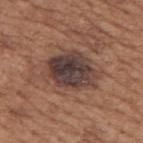Captured during whole-body skin photography for melanoma surveillance; the lesion was not biopsied. A male patient, aged 63–67. From the upper back. The recorded lesion diameter is about 6 mm. This image is a 15 mm lesion crop taken from a total-body photograph.